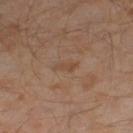{"biopsy_status": "not biopsied; imaged during a skin examination", "image": {"source": "total-body photography crop", "field_of_view_mm": 15}, "patient": {"sex": "male", "age_approx": 30}, "site": "leg", "automated_metrics": {"cielab_L": 45, "cielab_a": 16, "cielab_b": 28, "vs_skin_darker_L": 5.0}, "lesion_size": {"long_diameter_mm_approx": 3.0}}